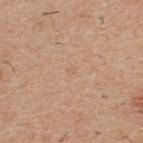Case summary:
– biopsy status: imaged on a skin check; not biopsied